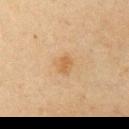Case summary:
- follow-up — no biopsy performed (imaged during a skin exam)
- illumination — cross-polarized
- acquisition — ~15 mm crop, total-body skin-cancer survey
- body site — the chest
- patient — male, about 65 years old